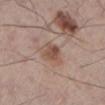Recorded during total-body skin imaging; not selected for excision or biopsy.
On the left lower leg.
A male patient aged 58–62.
A lesion tile, about 15 mm wide, cut from a 3D total-body photograph.
The lesion's longest dimension is about 3 mm.